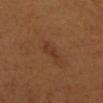biopsy_status: not biopsied; imaged during a skin examination
patient:
  sex: male
  age_approx: 65
image:
  source: total-body photography crop
  field_of_view_mm: 15
site: head or neck
lighting: cross-polarized